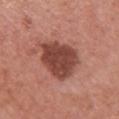• biopsy status · total-body-photography surveillance lesion; no biopsy
• imaging modality · 15 mm crop, total-body photography
• lesion size · about 6 mm
• subject · female, roughly 60 years of age
• illumination · white-light
• body site · the upper back